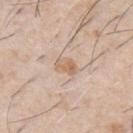notes = no biopsy performed (imaged during a skin exam)
imaging modality = ~15 mm tile from a whole-body skin photo
lighting = white-light illumination
subject = male, aged 58 to 62
anatomic site = the chest